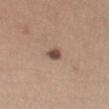biopsy_status: not biopsied; imaged during a skin examination
site: left thigh
patient:
  sex: female
  age_approx: 45
image:
  source: total-body photography crop
  field_of_view_mm: 15
lighting: white-light
lesion_size:
  long_diameter_mm_approx: 2.0